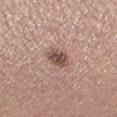Context: This image is a 15 mm lesion crop taken from a total-body photograph. The lesion is located on the left lower leg. The patient is a male aged 38 to 42.